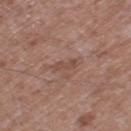Findings:
- notes · no biopsy performed (imaged during a skin exam)
- patient · male, aged approximately 60
- anatomic site · the right thigh
- acquisition · total-body-photography crop, ~15 mm field of view